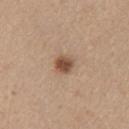The lesion was tiled from a total-body skin photograph and was not biopsied. The patient is a female aged 43–47. A close-up tile cropped from a whole-body skin photograph, about 15 mm across. The lesion is located on the right upper arm.